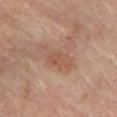Recorded during total-body skin imaging; not selected for excision or biopsy.
The lesion is located on the right leg.
Captured under cross-polarized illumination.
A close-up tile cropped from a whole-body skin photograph, about 15 mm across.
A male subject, in their 60s.